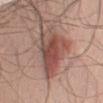Impression: Captured during whole-body skin photography for melanoma surveillance; the lesion was not biopsied. Acquisition and patient details: The lesion-visualizer software estimated a lesion area of about 20 mm² and a shape-asymmetry score of about 0.4 (0 = symmetric). And it measured a mean CIELAB color near L≈49 a*≈22 b*≈24 and a normalized border contrast of about 8.5. The software also gave a border-irregularity rating of about 4.5/10 and internal color variation of about 7 on a 0–10 scale. The analysis additionally found a classifier nevus-likeness of about 45/100 and a detector confidence of about 95 out of 100 that the crop contains a lesion. A lesion tile, about 15 mm wide, cut from a 3D total-body photograph. About 7.5 mm across. A male subject approximately 40 years of age. Captured under white-light illumination. On the left upper arm.